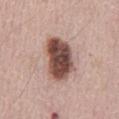The lesion was photographed on a routine skin check and not biopsied; there is no pathology result. A 15 mm crop from a total-body photograph taken for skin-cancer surveillance. The lesion's longest dimension is about 6 mm. The lesion is on the abdomen. The subject is a male aged 63–67.